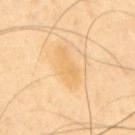{"biopsy_status": "not biopsied; imaged during a skin examination", "patient": {"sex": "male", "age_approx": 40}, "lighting": "cross-polarized", "image": {"source": "total-body photography crop", "field_of_view_mm": 15}, "lesion_size": {"long_diameter_mm_approx": 5.0}, "site": "upper back", "automated_metrics": {"cielab_L": 75, "cielab_a": 18, "cielab_b": 46, "vs_skin_darker_L": 6.0, "vs_skin_contrast_norm": 5.0, "border_irregularity_0_10": 3.5, "color_variation_0_10": 2.0, "peripheral_color_asymmetry": 0.5}}